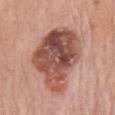<case>
  <biopsy_status>not biopsied; imaged during a skin examination</biopsy_status>
  <image>
    <source>total-body photography crop</source>
    <field_of_view_mm>15</field_of_view_mm>
  </image>
  <patient>
    <sex>male</sex>
    <age_approx>80</age_approx>
  </patient>
  <lesion_size>
    <long_diameter_mm_approx>9.0</long_diameter_mm_approx>
  </lesion_size>
  <site>chest</site>
  <lighting>white-light</lighting>
</case>